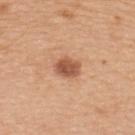The lesion was photographed on a routine skin check and not biopsied; there is no pathology result. A female subject aged approximately 30. A 15 mm close-up extracted from a 3D total-body photography capture. From the upper back.